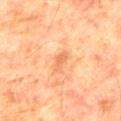<record>
  <biopsy_status>not biopsied; imaged during a skin examination</biopsy_status>
  <lesion_size>
    <long_diameter_mm_approx>3.0</long_diameter_mm_approx>
  </lesion_size>
  <automated_metrics>
    <border_irregularity_0_10>3.5</border_irregularity_0_10>
    <color_variation_0_10>0.5</color_variation_0_10>
    <peripheral_color_asymmetry>0.0</peripheral_color_asymmetry>
    <lesion_detection_confidence_0_100>100</lesion_detection_confidence_0_100>
  </automated_metrics>
  <image>
    <source>total-body photography crop</source>
    <field_of_view_mm>15</field_of_view_mm>
  </image>
  <patient>
    <sex>male</sex>
    <age_approx>75</age_approx>
  </patient>
  <site>mid back</site>
  <lighting>cross-polarized</lighting>
</record>